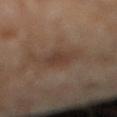Captured during whole-body skin photography for melanoma surveillance; the lesion was not biopsied.
The subject is a male about 70 years old.
This is a cross-polarized tile.
This image is a 15 mm lesion crop taken from a total-body photograph.
The lesion is located on the right lower leg.
The lesion's longest dimension is about 3 mm.